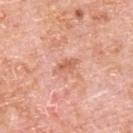Case summary:
- notes · no biopsy performed (imaged during a skin exam)
- diameter · ~3 mm (longest diameter)
- automated metrics · an area of roughly 3.5 mm², an outline eccentricity of about 0.85 (0 = round, 1 = elongated), and a symmetry-axis asymmetry near 0.25; a nevus-likeness score of about 0/100
- image · total-body-photography crop, ~15 mm field of view
- location · the upper back
- lighting · white-light
- patient · male, roughly 80 years of age